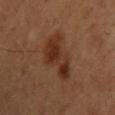follow-up: total-body-photography surveillance lesion; no biopsy
image: 15 mm crop, total-body photography
body site: the upper back
diameter: about 5.5 mm
automated metrics: an automated nevus-likeness rating near 55 out of 100 and a lesion-detection confidence of about 100/100
lighting: cross-polarized
subject: male, aged approximately 55